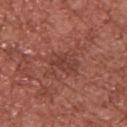{"patient": {"sex": "male", "age_approx": 45}, "site": "left upper arm", "image": {"source": "total-body photography crop", "field_of_view_mm": 15}}